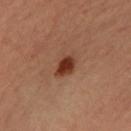{"biopsy_status": "not biopsied; imaged during a skin examination", "site": "left forearm", "lighting": "cross-polarized", "image": {"source": "total-body photography crop", "field_of_view_mm": 15}, "patient": {"sex": "female", "age_approx": 35}, "lesion_size": {"long_diameter_mm_approx": 3.0}}